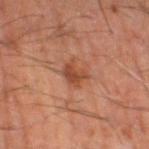Q: Was a biopsy performed?
A: imaged on a skin check; not biopsied
Q: How large is the lesion?
A: ≈3 mm
Q: How was this image acquired?
A: ~15 mm tile from a whole-body skin photo
Q: Lesion location?
A: the leg
Q: What are the patient's age and sex?
A: male, aged approximately 55